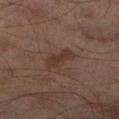No biopsy was performed on this lesion — it was imaged during a full skin examination and was not determined to be concerning. The lesion is located on the right lower leg. Captured under cross-polarized illumination. Automated tile analysis of the lesion measured a lesion color around L≈33 a*≈17 b*≈23 in CIELAB and a lesion-to-skin contrast of about 6.5 (normalized; higher = more distinct). The analysis additionally found a border-irregularity index near 4/10 and a within-lesion color-variation index near 2.5/10. A male subject, aged approximately 65. A 15 mm crop from a total-body photograph taken for skin-cancer surveillance. Approximately 3.5 mm at its widest.